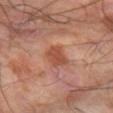Part of a total-body skin-imaging series; this lesion was reviewed on a skin check and was not flagged for biopsy. The recorded lesion diameter is about 3.5 mm. A male patient aged approximately 65. On the left forearm. This image is a 15 mm lesion crop taken from a total-body photograph.